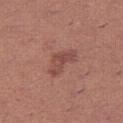Q: Was this lesion biopsied?
A: catalogued during a skin exam; not biopsied
Q: What kind of image is this?
A: ~15 mm tile from a whole-body skin photo
Q: Who is the patient?
A: female, about 25 years old
Q: Lesion location?
A: the right thigh